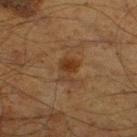The lesion was photographed on a routine skin check and not biopsied; there is no pathology result. A roughly 15 mm field-of-view crop from a total-body skin photograph. The tile uses cross-polarized illumination. A male patient, approximately 60 years of age. On the right thigh. Automated tile analysis of the lesion measured a classifier nevus-likeness of about 40/100 and a lesion-detection confidence of about 100/100. The lesion's longest dimension is about 3.5 mm.